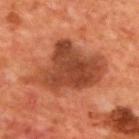| feature | finding |
|---|---|
| notes | imaged on a skin check; not biopsied |
| tile lighting | cross-polarized |
| image source | total-body-photography crop, ~15 mm field of view |
| patient | male, aged around 70 |
| automated metrics | a mean CIELAB color near L≈44 a*≈29 b*≈35 and a lesion-to-skin contrast of about 9.5 (normalized; higher = more distinct); a border-irregularity index near 5/10 and peripheral color asymmetry of about 1.5; an automated nevus-likeness rating near 0 out of 100 and a detector confidence of about 100 out of 100 that the crop contains a lesion |
| body site | the back |